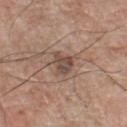The lesion was photographed on a routine skin check and not biopsied; there is no pathology result.
The lesion-visualizer software estimated a lesion area of about 5 mm² and an eccentricity of roughly 0.55. And it measured a lesion color around L≈47 a*≈17 b*≈23 in CIELAB, a lesion–skin lightness drop of about 10, and a lesion-to-skin contrast of about 8 (normalized; higher = more distinct).
About 2.5 mm across.
Imaged with white-light lighting.
On the chest.
A roughly 15 mm field-of-view crop from a total-body skin photograph.
A male patient, roughly 75 years of age.